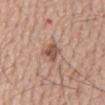Case summary:
- follow-up — imaged on a skin check; not biopsied
- illumination — white-light
- acquisition — ~15 mm crop, total-body skin-cancer survey
- anatomic site — the mid back
- size — about 3 mm
- subject — male, aged 63–67
- automated lesion analysis — a border-irregularity index near 4/10, a within-lesion color-variation index near 2.5/10, and peripheral color asymmetry of about 1